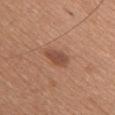biopsy status: total-body-photography surveillance lesion; no biopsy | image: total-body-photography crop, ~15 mm field of view | illumination: white-light illumination | body site: the chest | subject: female, in their mid- to late 50s.